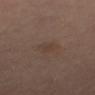site: right thigh
patient:
  sex: male
  age_approx: 65
image:
  source: total-body photography crop
  field_of_view_mm: 15